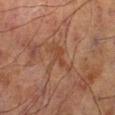Impression:
No biopsy was performed on this lesion — it was imaged during a full skin examination and was not determined to be concerning.